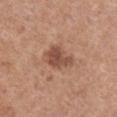Recorded during total-body skin imaging; not selected for excision or biopsy.
Imaged with white-light lighting.
On the front of the torso.
Automated image analysis of the tile measured an average lesion color of about L≈50 a*≈22 b*≈29 (CIELAB), about 11 CIELAB-L* units darker than the surrounding skin, and a normalized lesion–skin contrast near 7.5. And it measured an automated nevus-likeness rating near 20 out of 100 and a lesion-detection confidence of about 100/100.
The recorded lesion diameter is about 3.5 mm.
A 15 mm close-up extracted from a 3D total-body photography capture.
A female patient, about 65 years old.